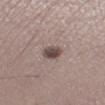follow-up: no biopsy performed (imaged during a skin exam)
acquisition: ~15 mm tile from a whole-body skin photo
patient: male, roughly 50 years of age
location: the leg
lighting: white-light illumination
lesion diameter: ~2.5 mm (longest diameter)